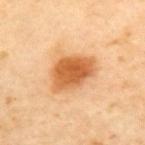Imaged during a routine full-body skin examination; the lesion was not biopsied and no histopathology is available. A male subject approximately 65 years of age. Measured at roughly 5 mm in maximum diameter. A region of skin cropped from a whole-body photographic capture, roughly 15 mm wide. From the upper back.